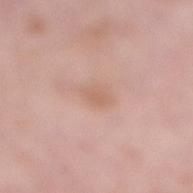workup — no biopsy performed (imaged during a skin exam)
body site — the right lower leg
illumination — white-light
image — total-body-photography crop, ~15 mm field of view
patient — female, roughly 70 years of age
diameter — about 2.5 mm